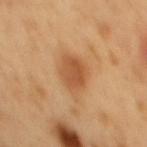follow-up — total-body-photography surveillance lesion; no biopsy
imaging modality — ~15 mm tile from a whole-body skin photo
subject — male, aged 48 to 52
body site — the mid back